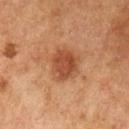| feature | finding |
|---|---|
| workup | imaged on a skin check; not biopsied |
| acquisition | ~15 mm crop, total-body skin-cancer survey |
| subject | female, in their 60s |
| automated metrics | a shape eccentricity near 0.6; a within-lesion color-variation index near 2.5/10 and peripheral color asymmetry of about 1; an automated nevus-likeness rating near 95 out of 100 and lesion-presence confidence of about 100/100 |
| tile lighting | cross-polarized illumination |
| site | the arm |